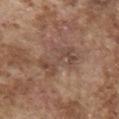{
  "biopsy_status": "not biopsied; imaged during a skin examination",
  "image": {
    "source": "total-body photography crop",
    "field_of_view_mm": 15
  },
  "site": "front of the torso",
  "lesion_size": {
    "long_diameter_mm_approx": 6.0
  },
  "patient": {
    "sex": "male",
    "age_approx": 75
  },
  "lighting": "white-light"
}